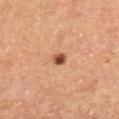<lesion>
  <biopsy_status>not biopsied; imaged during a skin examination</biopsy_status>
  <image>
    <source>total-body photography crop</source>
    <field_of_view_mm>15</field_of_view_mm>
  </image>
  <site>right lower leg</site>
  <lesion_size>
    <long_diameter_mm_approx>2.0</long_diameter_mm_approx>
  </lesion_size>
  <patient>
    <sex>male</sex>
    <age_approx>65</age_approx>
  </patient>
</lesion>